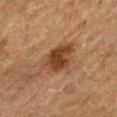Image and clinical context: Captured under cross-polarized illumination. The total-body-photography lesion software estimated an area of roughly 12 mm², an outline eccentricity of about 0.65 (0 = round, 1 = elongated), and a symmetry-axis asymmetry near 0.25. The analysis additionally found a mean CIELAB color near L≈34 a*≈18 b*≈27, a lesion–skin lightness drop of about 10, and a lesion-to-skin contrast of about 9 (normalized; higher = more distinct). The software also gave border irregularity of about 2.5 on a 0–10 scale, internal color variation of about 4.5 on a 0–10 scale, and a peripheral color-asymmetry measure near 1.5. This image is a 15 mm lesion crop taken from a total-body photograph. The subject is a male in their mid-70s. The recorded lesion diameter is about 4.5 mm. On the leg.